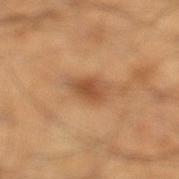Captured during whole-body skin photography for melanoma surveillance; the lesion was not biopsied.
An algorithmic analysis of the crop reported an area of roughly 7 mm², a shape eccentricity near 0.9, and a symmetry-axis asymmetry near 0.25. The software also gave an average lesion color of about L≈52 a*≈22 b*≈36 (CIELAB), roughly 11 lightness units darker than nearby skin, and a normalized lesion–skin contrast near 7.5. It also reported an automated nevus-likeness rating near 55 out of 100.
A 15 mm crop from a total-body photograph taken for skin-cancer surveillance.
The patient is a male aged 38–42.
On the left lower leg.